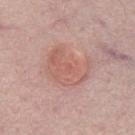patient=male, approximately 65 years of age
anatomic site=the chest
automated lesion analysis=roughly 7 lightness units darker than nearby skin and a normalized border contrast of about 5; a peripheral color-asymmetry measure near 1; a classifier nevus-likeness of about 25/100 and a detector confidence of about 100 out of 100 that the crop contains a lesion
image=15 mm crop, total-body photography
lighting=white-light
diameter=about 5.5 mm